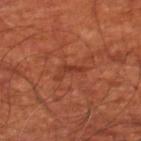No biopsy was performed on this lesion — it was imaged during a full skin examination and was not determined to be concerning. A subject in their mid- to late 60s. A close-up tile cropped from a whole-body skin photograph, about 15 mm across. Captured under cross-polarized illumination. On the right lower leg. Measured at roughly 3.5 mm in maximum diameter.